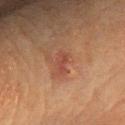Assessment: Imaged during a routine full-body skin examination; the lesion was not biopsied and no histopathology is available. Background: The lesion is located on the head or neck. A female patient approximately 80 years of age. A lesion tile, about 15 mm wide, cut from a 3D total-body photograph. Automated tile analysis of the lesion measured an area of roughly 3 mm², an eccentricity of roughly 0.9, and a symmetry-axis asymmetry near 0.25. The software also gave an average lesion color of about L≈40 a*≈23 b*≈27 (CIELAB), a lesion–skin lightness drop of about 7, and a normalized lesion–skin contrast near 6. The analysis additionally found a border-irregularity index near 3/10, internal color variation of about 0 on a 0–10 scale, and a peripheral color-asymmetry measure near 0. And it measured an automated nevus-likeness rating near 15 out of 100 and a lesion-detection confidence of about 100/100. The lesion's longest dimension is about 2.5 mm.